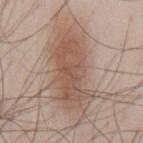| field | value |
|---|---|
| biopsy status | imaged on a skin check; not biopsied |
| image source | total-body-photography crop, ~15 mm field of view |
| patient | male, aged 43–47 |
| site | the chest |
| diameter | ≈11 mm |
| automated metrics | a lesion area of about 34 mm², a shape eccentricity near 0.9, and a symmetry-axis asymmetry near 0.25; radial color variation of about 1.5 |
| illumination | white-light illumination |